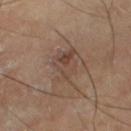biopsy_status: not biopsied; imaged during a skin examination
lesion_size:
  long_diameter_mm_approx: 4.5
patient:
  sex: male
  age_approx: 65
site: left thigh
automated_metrics:
  eccentricity: 0.8
  shape_asymmetry: 0.35
image:
  source: total-body photography crop
  field_of_view_mm: 15